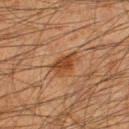Impression: The lesion was tiled from a total-body skin photograph and was not biopsied. Background: An algorithmic analysis of the crop reported an area of roughly 5.5 mm², an eccentricity of roughly 0.75, and a symmetry-axis asymmetry near 0.3. The analysis additionally found border irregularity of about 3 on a 0–10 scale, a color-variation rating of about 2.5/10, and peripheral color asymmetry of about 0.5. It also reported an automated nevus-likeness rating near 80 out of 100 and a detector confidence of about 100 out of 100 that the crop contains a lesion. The lesion is on the left thigh. A male subject aged 33 to 37. Captured under cross-polarized illumination. A 15 mm close-up extracted from a 3D total-body photography capture.